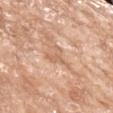The lesion was photographed on a routine skin check and not biopsied; there is no pathology result. Captured under white-light illumination. Located on the left upper arm. A female patient, aged around 75. About 3.5 mm across. A region of skin cropped from a whole-body photographic capture, roughly 15 mm wide.